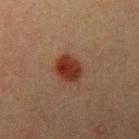No biopsy was performed on this lesion — it was imaged during a full skin examination and was not determined to be concerning.
The patient is a male aged 38 to 42.
A lesion tile, about 15 mm wide, cut from a 3D total-body photograph.
The lesion is located on the left upper arm.